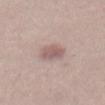Assessment: The lesion was photographed on a routine skin check and not biopsied; there is no pathology result. Image and clinical context: Longest diameter approximately 3 mm. Automated image analysis of the tile measured a shape eccentricity near 0.7. The analysis additionally found roughly 10 lightness units darker than nearby skin. And it measured a within-lesion color-variation index near 3/10 and peripheral color asymmetry of about 1. The analysis additionally found an automated nevus-likeness rating near 65 out of 100 and a detector confidence of about 100 out of 100 that the crop contains a lesion. Cropped from a total-body skin-imaging series; the visible field is about 15 mm. The subject is a male aged 28–32. The tile uses white-light illumination.